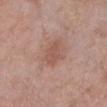notes: catalogued during a skin exam; not biopsied